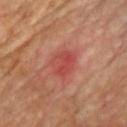• follow-up — catalogued during a skin exam; not biopsied